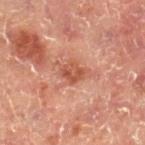{"biopsy_status": "not biopsied; imaged during a skin examination", "site": "leg", "lighting": "cross-polarized", "patient": {"sex": "male", "age_approx": 50}, "image": {"source": "total-body photography crop", "field_of_view_mm": 15}}